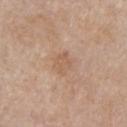notes: imaged on a skin check; not biopsied | diameter: ~2.5 mm (longest diameter) | patient: male, aged 58 to 62 | body site: the front of the torso | automated lesion analysis: an eccentricity of roughly 0.55 and two-axis asymmetry of about 0.3; an average lesion color of about L≈58 a*≈19 b*≈31 (CIELAB); a classifier nevus-likeness of about 0/100 and lesion-presence confidence of about 100/100 | acquisition: ~15 mm tile from a whole-body skin photo.